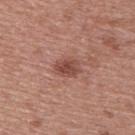Imaged during a routine full-body skin examination; the lesion was not biopsied and no histopathology is available. The patient is a male aged 53 to 57. Imaged with white-light lighting. This image is a 15 mm lesion crop taken from a total-body photograph. Approximately 3 mm at its widest. The lesion is on the back.